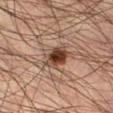This lesion was catalogued during total-body skin photography and was not selected for biopsy.
Captured under cross-polarized illumination.
Measured at roughly 4.5 mm in maximum diameter.
A lesion tile, about 15 mm wide, cut from a 3D total-body photograph.
The patient is a male approximately 45 years of age.
The lesion is located on the right lower leg.